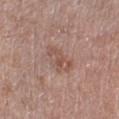Notes:
– follow-up · imaged on a skin check; not biopsied
– diameter · ≈4 mm
– image source · ~15 mm crop, total-body skin-cancer survey
– subject · female, in their mid- to late 70s
– location · the left thigh
– automated lesion analysis · an automated nevus-likeness rating near 0 out of 100 and a detector confidence of about 100 out of 100 that the crop contains a lesion
– illumination · white-light illumination